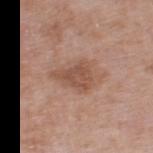A 15 mm close-up extracted from a 3D total-body photography capture. Located on the left thigh. Imaged with white-light lighting. About 5 mm across. A male patient, roughly 80 years of age.